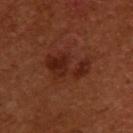{
  "patient": {
    "sex": "female",
    "age_approx": 55
  },
  "image": {
    "source": "total-body photography crop",
    "field_of_view_mm": 15
  },
  "lesion_size": {
    "long_diameter_mm_approx": 5.0
  },
  "site": "upper back",
  "lighting": "cross-polarized"
}